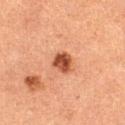No biopsy was performed on this lesion — it was imaged during a full skin examination and was not determined to be concerning.
This is a cross-polarized tile.
Measured at roughly 2.5 mm in maximum diameter.
An algorithmic analysis of the crop reported an eccentricity of roughly 0.35 and a symmetry-axis asymmetry near 0.2.
A female patient aged 58 to 62.
A 15 mm close-up extracted from a 3D total-body photography capture.
From the left thigh.